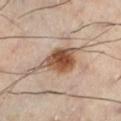| field | value |
|---|---|
| biopsy status | imaged on a skin check; not biopsied |
| diameter | ≈5.5 mm |
| site | the left lower leg |
| image-analysis metrics | a within-lesion color-variation index near 7/10 and a peripheral color-asymmetry measure near 2 |
| imaging modality | 15 mm crop, total-body photography |
| subject | male, aged around 40 |